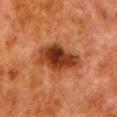Assessment: Recorded during total-body skin imaging; not selected for excision or biopsy. Background: The lesion is located on the left lower leg. The recorded lesion diameter is about 6.5 mm. Cropped from a whole-body photographic skin survey; the tile spans about 15 mm. A male patient aged approximately 80.